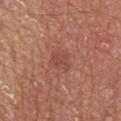| field | value |
|---|---|
| follow-up | imaged on a skin check; not biopsied |
| automated metrics | a shape eccentricity near 0.75 and two-axis asymmetry of about 0.3 |
| image source | ~15 mm crop, total-body skin-cancer survey |
| size | ~3 mm (longest diameter) |
| anatomic site | the mid back |
| patient | male, approximately 65 years of age |